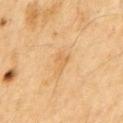Acquisition and patient details: The lesion is located on the chest. Longest diameter approximately 3 mm. The tile uses cross-polarized illumination. A male patient, roughly 70 years of age. The total-body-photography lesion software estimated a footprint of about 3.5 mm² and an outline eccentricity of about 0.85 (0 = round, 1 = elongated). It also reported an average lesion color of about L≈63 a*≈19 b*≈42 (CIELAB), about 6 CIELAB-L* units darker than the surrounding skin, and a normalized lesion–skin contrast near 5. And it measured a border-irregularity index near 5/10, a within-lesion color-variation index near 1/10, and a peripheral color-asymmetry measure near 0.5. A 15 mm close-up extracted from a 3D total-body photography capture.